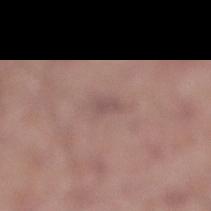Part of a total-body skin-imaging series; this lesion was reviewed on a skin check and was not flagged for biopsy.
Measured at roughly 2.5 mm in maximum diameter.
This is a white-light tile.
A male patient, aged around 55.
The lesion is located on the right lower leg.
This image is a 15 mm lesion crop taken from a total-body photograph.